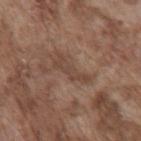{
  "patient": {
    "sex": "male",
    "age_approx": 75
  },
  "image": {
    "source": "total-body photography crop",
    "field_of_view_mm": 15
  },
  "site": "chest"
}